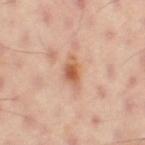This lesion was catalogued during total-body skin photography and was not selected for biopsy. A male subject about 55 years old. On the left thigh. A 15 mm close-up tile from a total-body photography series done for melanoma screening. Longest diameter approximately 3.5 mm.